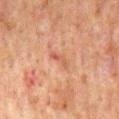<case>
  <biopsy_status>not biopsied; imaged during a skin examination</biopsy_status>
  <lighting>cross-polarized</lighting>
  <lesion_size>
    <long_diameter_mm_approx>3.0</long_diameter_mm_approx>
  </lesion_size>
  <image>
    <source>total-body photography crop</source>
    <field_of_view_mm>15</field_of_view_mm>
  </image>
  <site>back</site>
  <patient>
    <sex>male</sex>
    <age_approx>60</age_approx>
  </patient>
</case>